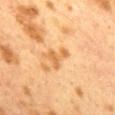Recorded during total-body skin imaging; not selected for excision or biopsy.
From the mid back.
Cropped from a total-body skin-imaging series; the visible field is about 15 mm.
An algorithmic analysis of the crop reported an eccentricity of roughly 0.7 and a shape-asymmetry score of about 0.65 (0 = symmetric). It also reported an average lesion color of about L≈54 a*≈20 b*≈39 (CIELAB) and about 8 CIELAB-L* units darker than the surrounding skin. The software also gave internal color variation of about 0.5 on a 0–10 scale and a peripheral color-asymmetry measure near 0.5. And it measured a classifier nevus-likeness of about 0/100.
Measured at roughly 3 mm in maximum diameter.
A female subject roughly 40 years of age.